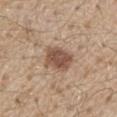Image and clinical context: This image is a 15 mm lesion crop taken from a total-body photograph. The subject is a male aged approximately 70. On the chest.